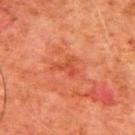Located on the upper back. A male patient, in their 80s. Imaged with cross-polarized lighting. Measured at roughly 3 mm in maximum diameter. Cropped from a total-body skin-imaging series; the visible field is about 15 mm. An algorithmic analysis of the crop reported a footprint of about 3 mm², an eccentricity of roughly 0.8, and two-axis asymmetry of about 0.5. It also reported a mean CIELAB color near L≈40 a*≈31 b*≈33, about 6 CIELAB-L* units darker than the surrounding skin, and a normalized border contrast of about 5. The software also gave a border-irregularity rating of about 5.5/10 and a peripheral color-asymmetry measure near 0. It also reported a nevus-likeness score of about 0/100 and a lesion-detection confidence of about 100/100.